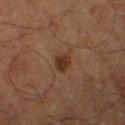Clinical impression: Part of a total-body skin-imaging series; this lesion was reviewed on a skin check and was not flagged for biopsy. Background: An algorithmic analysis of the crop reported a footprint of about 4 mm², a shape eccentricity near 0.75, and a symmetry-axis asymmetry near 0.2. The analysis additionally found an average lesion color of about L≈26 a*≈17 b*≈24 (CIELAB), about 8 CIELAB-L* units darker than the surrounding skin, and a lesion-to-skin contrast of about 9 (normalized; higher = more distinct). The software also gave a within-lesion color-variation index near 1.5/10 and a peripheral color-asymmetry measure near 0.5. The software also gave a classifier nevus-likeness of about 85/100. A male patient, about 70 years old. The tile uses cross-polarized illumination. The recorded lesion diameter is about 2.5 mm. A 15 mm close-up extracted from a 3D total-body photography capture. Located on the right thigh.